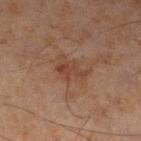Assessment:
Recorded during total-body skin imaging; not selected for excision or biopsy.
Context:
The tile uses cross-polarized illumination. A roughly 15 mm field-of-view crop from a total-body skin photograph. About 3.5 mm across. The lesion is located on the left lower leg. The total-body-photography lesion software estimated a mean CIELAB color near L≈33 a*≈18 b*≈24 and a lesion-to-skin contrast of about 6 (normalized; higher = more distinct). And it measured a border-irregularity rating of about 5/10, a color-variation rating of about 2/10, and radial color variation of about 0.5. The analysis additionally found an automated nevus-likeness rating near 0 out of 100 and a lesion-detection confidence of about 100/100. The patient is a male aged 43 to 47.